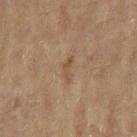This lesion was catalogued during total-body skin photography and was not selected for biopsy. About 3 mm across. This is a cross-polarized tile. A female patient, aged 78–82. On the right arm. A region of skin cropped from a whole-body photographic capture, roughly 15 mm wide. An algorithmic analysis of the crop reported an average lesion color of about L≈45 a*≈15 b*≈28 (CIELAB), roughly 6 lightness units darker than nearby skin, and a normalized border contrast of about 5.5. The analysis additionally found a border-irregularity index near 6/10 and a within-lesion color-variation index near 0/10.